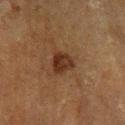The lesion was tiled from a total-body skin photograph and was not biopsied. From the left lower leg. A region of skin cropped from a whole-body photographic capture, roughly 15 mm wide. A male patient aged 58 to 62. An algorithmic analysis of the crop reported an area of roughly 5.5 mm² and a shape-asymmetry score of about 0.3 (0 = symmetric). And it measured about 8 CIELAB-L* units darker than the surrounding skin and a normalized border contrast of about 9.5. The software also gave a nevus-likeness score of about 65/100 and a detector confidence of about 100 out of 100 that the crop contains a lesion. The tile uses cross-polarized illumination.